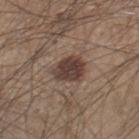{"biopsy_status": "not biopsied; imaged during a skin examination", "image": {"source": "total-body photography crop", "field_of_view_mm": 15}, "lighting": "white-light", "patient": {"sex": "male", "age_approx": 45}, "site": "right lower leg", "lesion_size": {"long_diameter_mm_approx": 3.5}}